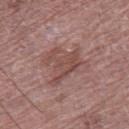biopsy status: no biopsy performed (imaged during a skin exam)
anatomic site: the right thigh
image source: ~15 mm tile from a whole-body skin photo
patient: male, approximately 75 years of age
size: about 5 mm